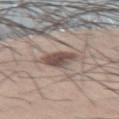Impression: No biopsy was performed on this lesion — it was imaged during a full skin examination and was not determined to be concerning. Background: On the right thigh. The lesion's longest dimension is about 3.5 mm. The tile uses white-light illumination. A male patient in their 60s. Automated image analysis of the tile measured a mean CIELAB color near L≈49 a*≈14 b*≈20, about 13 CIELAB-L* units darker than the surrounding skin, and a normalized border contrast of about 9.5. Cropped from a total-body skin-imaging series; the visible field is about 15 mm.